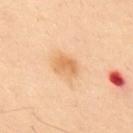Captured during whole-body skin photography for melanoma surveillance; the lesion was not biopsied.
The patient is a male in their 50s.
A roughly 15 mm field-of-view crop from a total-body skin photograph.
The lesion is on the back.